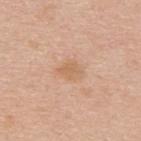<tbp_lesion>
  <biopsy_status>not biopsied; imaged during a skin examination</biopsy_status>
  <patient>
    <sex>male</sex>
    <age_approx>35</age_approx>
  </patient>
  <lesion_size>
    <long_diameter_mm_approx>2.5</long_diameter_mm_approx>
  </lesion_size>
  <image>
    <source>total-body photography crop</source>
    <field_of_view_mm>15</field_of_view_mm>
  </image>
  <automated_metrics>
    <lesion_detection_confidence_0_100>100</lesion_detection_confidence_0_100>
  </automated_metrics>
  <site>upper back</site>
  <lighting>white-light</lighting>
</tbp_lesion>